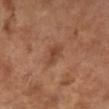- notes · total-body-photography surveillance lesion; no biopsy
- site · the right lower leg
- automated metrics · an area of roughly 4.5 mm² and an outline eccentricity of about 0.85 (0 = round, 1 = elongated); an average lesion color of about L≈44 a*≈22 b*≈31 (CIELAB), roughly 8 lightness units darker than nearby skin, and a lesion-to-skin contrast of about 6 (normalized; higher = more distinct); a border-irregularity rating of about 2.5/10, internal color variation of about 2 on a 0–10 scale, and radial color variation of about 0.5; an automated nevus-likeness rating near 0 out of 100 and a detector confidence of about 100 out of 100 that the crop contains a lesion
- subject · male, aged 63 to 67
- illumination · cross-polarized illumination
- acquisition · total-body-photography crop, ~15 mm field of view